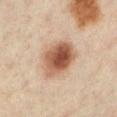workup: no biopsy performed (imaged during a skin exam) | automated lesion analysis: border irregularity of about 1.5 on a 0–10 scale, a within-lesion color-variation index near 6.5/10, and radial color variation of about 2; a classifier nevus-likeness of about 100/100 and a detector confidence of about 100 out of 100 that the crop contains a lesion | subject: female, in their mid- to late 40s | tile lighting: cross-polarized | acquisition: ~15 mm crop, total-body skin-cancer survey | body site: the leg | size: ≈4.5 mm.